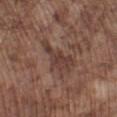  biopsy_status: not biopsied; imaged during a skin examination
  image:
    source: total-body photography crop
    field_of_view_mm: 15
  patient:
    sex: male
    age_approx: 75
  site: left lower leg